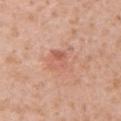notes — imaged on a skin check; not biopsied | diameter — ≈3.5 mm | body site — the left upper arm | subject — female, aged around 40 | imaging modality — ~15 mm tile from a whole-body skin photo.